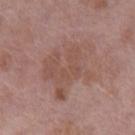Case summary:
- biopsy status · catalogued during a skin exam; not biopsied
- automated metrics · a shape eccentricity near 0.65 and a symmetry-axis asymmetry near 0.4; an average lesion color of about L≈51 a*≈20 b*≈24 (CIELAB), roughly 6 lightness units darker than nearby skin, and a normalized lesion–skin contrast near 5; peripheral color asymmetry of about 1
- tile lighting · white-light illumination
- lesion diameter · about 7 mm
- location · the left lower leg
- image source · 15 mm crop, total-body photography
- subject · female, about 70 years old